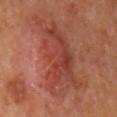This lesion was catalogued during total-body skin photography and was not selected for biopsy. Approximately 11 mm at its widest. Imaged with cross-polarized lighting. A region of skin cropped from a whole-body photographic capture, roughly 15 mm wide. The total-body-photography lesion software estimated a shape-asymmetry score of about 0.4 (0 = symmetric). And it measured an automated nevus-likeness rating near 0 out of 100 and a detector confidence of about 100 out of 100 that the crop contains a lesion. Located on the mid back. The patient is a male approximately 65 years of age.